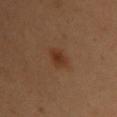Captured during whole-body skin photography for melanoma surveillance; the lesion was not biopsied.
Located on the left upper arm.
A female patient aged approximately 50.
A 15 mm close-up extracted from a 3D total-body photography capture.